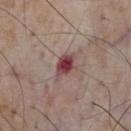The lesion was tiled from a total-body skin photograph and was not biopsied.
This image is a 15 mm lesion crop taken from a total-body photograph.
A male subject roughly 75 years of age.
Captured under white-light illumination.
On the chest.
Approximately 3.5 mm at its widest.